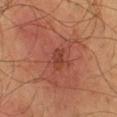Findings:
• biopsy status: total-body-photography surveillance lesion; no biopsy
• subject: male, about 55 years old
• lighting: cross-polarized
• anatomic site: the left lower leg
• imaging modality: ~15 mm tile from a whole-body skin photo
• lesion diameter: ≈2.5 mm